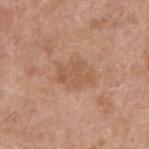| key | value |
|---|---|
| biopsy status | imaged on a skin check; not biopsied |
| lesion size | about 4 mm |
| subject | male, aged 58–62 |
| imaging modality | 15 mm crop, total-body photography |
| location | the arm |
| lighting | white-light illumination |